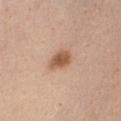Imaged during a routine full-body skin examination; the lesion was not biopsied and no histopathology is available.
The total-body-photography lesion software estimated a footprint of about 5 mm² and a symmetry-axis asymmetry near 0.25. It also reported an average lesion color of about L≈56 a*≈21 b*≈34 (CIELAB), roughly 13 lightness units darker than nearby skin, and a normalized lesion–skin contrast near 9. The software also gave border irregularity of about 2 on a 0–10 scale, internal color variation of about 2.5 on a 0–10 scale, and a peripheral color-asymmetry measure near 0.5.
The patient is a female about 40 years old.
The tile uses white-light illumination.
From the chest.
The lesion's longest dimension is about 3 mm.
A lesion tile, about 15 mm wide, cut from a 3D total-body photograph.